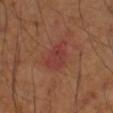biopsy status: no biopsy performed (imaged during a skin exam)
lighting: cross-polarized illumination
subject: male, in their mid- to late 40s
body site: the right forearm
imaging modality: total-body-photography crop, ~15 mm field of view
TBP lesion metrics: a shape eccentricity near 0.75 and a shape-asymmetry score of about 0.35 (0 = symmetric); a mean CIELAB color near L≈38 a*≈28 b*≈25 and a normalized lesion–skin contrast near 6; a border-irregularity index near 4/10 and a within-lesion color-variation index near 2.5/10; a classifier nevus-likeness of about 0/100 and lesion-presence confidence of about 100/100